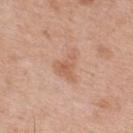Notes:
• notes · imaged on a skin check; not biopsied
• lesion size · ≈4 mm
• subject · male, in their mid-50s
• tile lighting · white-light illumination
• acquisition · total-body-photography crop, ~15 mm field of view
• body site · the upper back